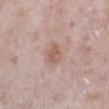The lesion was photographed on a routine skin check and not biopsied; there is no pathology result.
A male subject, in their 60s.
Measured at roughly 2.5 mm in maximum diameter.
The tile uses white-light illumination.
Automated tile analysis of the lesion measured a classifier nevus-likeness of about 20/100 and a lesion-detection confidence of about 100/100.
The lesion is located on the right lower leg.
This image is a 15 mm lesion crop taken from a total-body photograph.